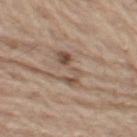Q: Lesion location?
A: the left thigh
Q: Lesion size?
A: about 5.5 mm
Q: Automated lesion metrics?
A: border irregularity of about 7.5 on a 0–10 scale, a color-variation rating of about 4.5/10, and a peripheral color-asymmetry measure near 1.5
Q: Patient demographics?
A: male, in their 70s
Q: How was this image acquired?
A: 15 mm crop, total-body photography